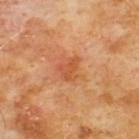Q: Was this lesion biopsied?
A: total-body-photography surveillance lesion; no biopsy
Q: Lesion size?
A: ≈3 mm
Q: Who is the patient?
A: male, approximately 60 years of age
Q: What is the anatomic site?
A: the chest
Q: How was this image acquired?
A: ~15 mm tile from a whole-body skin photo
Q: How was the tile lit?
A: cross-polarized